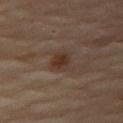biopsy status: catalogued during a skin exam; not biopsied
automated metrics: an outline eccentricity of about 0.55 (0 = round, 1 = elongated) and two-axis asymmetry of about 0.2; a classifier nevus-likeness of about 60/100 and lesion-presence confidence of about 100/100
imaging modality: ~15 mm tile from a whole-body skin photo
patient: female, in their 70s
lesion size: ~3 mm (longest diameter)
location: the right thigh
illumination: cross-polarized